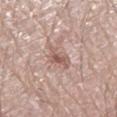Image and clinical context: The lesion-visualizer software estimated a footprint of about 5 mm², an outline eccentricity of about 0.85 (0 = round, 1 = elongated), and a symmetry-axis asymmetry near 0.35. The software also gave roughly 10 lightness units darker than nearby skin and a normalized lesion–skin contrast near 7. And it measured border irregularity of about 4.5 on a 0–10 scale and a within-lesion color-variation index near 3/10. The software also gave a classifier nevus-likeness of about 20/100 and a lesion-detection confidence of about 100/100. The tile uses white-light illumination. The lesion is located on the left leg. A male patient aged around 80. A close-up tile cropped from a whole-body skin photograph, about 15 mm across.